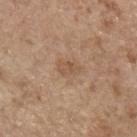Impression: Imaged during a routine full-body skin examination; the lesion was not biopsied and no histopathology is available. Acquisition and patient details: The lesion-visualizer software estimated a shape eccentricity near 0.75 and a symmetry-axis asymmetry near 0.3. And it measured border irregularity of about 3 on a 0–10 scale, internal color variation of about 2 on a 0–10 scale, and a peripheral color-asymmetry measure near 1. This is a white-light tile. The subject is a female in their mid- to late 60s. Approximately 3 mm at its widest. A roughly 15 mm field-of-view crop from a total-body skin photograph. The lesion is on the left forearm.